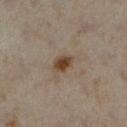Clinical impression: Imaged during a routine full-body skin examination; the lesion was not biopsied and no histopathology is available. Context: A female patient in their mid-30s. Located on the left lower leg. A region of skin cropped from a whole-body photographic capture, roughly 15 mm wide.